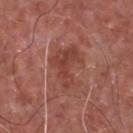| key | value |
|---|---|
| notes | total-body-photography surveillance lesion; no biopsy |
| acquisition | 15 mm crop, total-body photography |
| location | the front of the torso |
| patient | male, approximately 65 years of age |
| size | ≈5.5 mm |
| illumination | white-light |